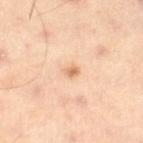Clinical impression: The lesion was photographed on a routine skin check and not biopsied; there is no pathology result. Background: The recorded lesion diameter is about 1.5 mm. A lesion tile, about 15 mm wide, cut from a 3D total-body photograph. This is a cross-polarized tile. The patient is a male aged 53 to 57. The lesion is on the right lower leg.